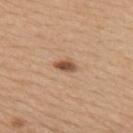Captured during whole-body skin photography for melanoma surveillance; the lesion was not biopsied.
The lesion is on the upper back.
A female subject aged approximately 30.
Imaged with white-light lighting.
A 15 mm close-up tile from a total-body photography series done for melanoma screening.
The lesion's longest dimension is about 2.5 mm.